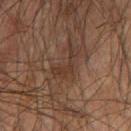Recorded during total-body skin imaging; not selected for excision or biopsy. A male patient, aged around 75. From the right forearm. Captured under cross-polarized illumination. Automated image analysis of the tile measured a lesion area of about 4.5 mm², an outline eccentricity of about 0.9 (0 = round, 1 = elongated), and a shape-asymmetry score of about 0.7 (0 = symmetric). A 15 mm crop from a total-body photograph taken for skin-cancer surveillance. About 4.5 mm across.